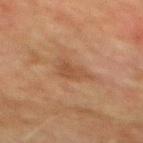biopsy status=total-body-photography surveillance lesion; no biopsy | imaging modality=~15 mm crop, total-body skin-cancer survey | illumination=cross-polarized | size=~4 mm (longest diameter) | site=the mid back | patient=male, aged approximately 75.